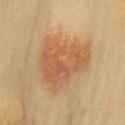No biopsy was performed on this lesion — it was imaged during a full skin examination and was not determined to be concerning.
A lesion tile, about 15 mm wide, cut from a 3D total-body photograph.
Longest diameter approximately 6.5 mm.
The tile uses cross-polarized illumination.
The lesion is on the chest.
A female subject aged approximately 60.